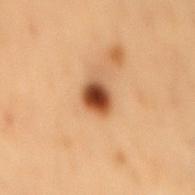Recorded during total-body skin imaging; not selected for excision or biopsy. Approximately 3 mm at its widest. Cropped from a whole-body photographic skin survey; the tile spans about 15 mm. The tile uses cross-polarized illumination. The lesion is located on the mid back. The subject is a male aged 53–57.